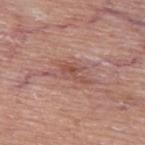<lesion>
<biopsy_status>not biopsied; imaged during a skin examination</biopsy_status>
<lesion_size>
  <long_diameter_mm_approx>5.5</long_diameter_mm_approx>
</lesion_size>
<patient>
  <sex>male</sex>
  <age_approx>80</age_approx>
</patient>
<lighting>white-light</lighting>
<site>back</site>
<image>
  <source>total-body photography crop</source>
  <field_of_view_mm>15</field_of_view_mm>
</image>
</lesion>